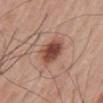Part of a total-body skin-imaging series; this lesion was reviewed on a skin check and was not flagged for biopsy. A 15 mm close-up extracted from a 3D total-body photography capture. Longest diameter approximately 4 mm. The patient is a male aged 68 to 72. From the mid back. Captured under white-light illumination.